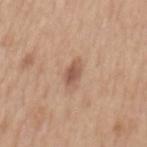No biopsy was performed on this lesion — it was imaged during a full skin examination and was not determined to be concerning. Measured at roughly 3 mm in maximum diameter. A region of skin cropped from a whole-body photographic capture, roughly 15 mm wide. The lesion is on the mid back. A female patient roughly 40 years of age.